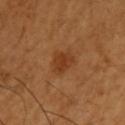The lesion was tiled from a total-body skin photograph and was not biopsied. A male subject aged 63 to 67. The tile uses cross-polarized illumination. The recorded lesion diameter is about 3 mm. An algorithmic analysis of the crop reported a footprint of about 5 mm² and a shape-asymmetry score of about 0.3 (0 = symmetric). And it measured a mean CIELAB color near L≈38 a*≈24 b*≈37 and a lesion-to-skin contrast of about 7 (normalized; higher = more distinct). The analysis additionally found border irregularity of about 2.5 on a 0–10 scale, internal color variation of about 2 on a 0–10 scale, and peripheral color asymmetry of about 0.5. The software also gave a nevus-likeness score of about 55/100 and a detector confidence of about 100 out of 100 that the crop contains a lesion. The lesion is located on the left upper arm. A 15 mm close-up extracted from a 3D total-body photography capture.